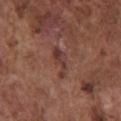patient=male, in their mid- to late 70s
acquisition=~15 mm crop, total-body skin-cancer survey
lesion diameter=~4 mm (longest diameter)
site=the chest